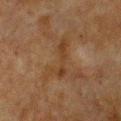  biopsy_status: not biopsied; imaged during a skin examination